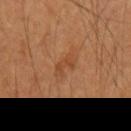{
  "biopsy_status": "not biopsied; imaged during a skin examination",
  "automated_metrics": {
    "area_mm2_approx": 4.5,
    "eccentricity": 0.85,
    "shape_asymmetry": 0.35,
    "vs_skin_darker_L": 6.0,
    "vs_skin_contrast_norm": 5.0,
    "nevus_likeness_0_100": 0,
    "lesion_detection_confidence_0_100": 100
  },
  "patient": {
    "sex": "male",
    "age_approx": 55
  },
  "image": {
    "source": "total-body photography crop",
    "field_of_view_mm": 15
  },
  "lighting": "cross-polarized",
  "lesion_size": {
    "long_diameter_mm_approx": 3.0
  },
  "site": "arm"
}